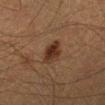| key | value |
|---|---|
| workup | no biopsy performed (imaged during a skin exam) |
| subject | male, aged approximately 40 |
| image-analysis metrics | a symmetry-axis asymmetry near 0.15; an average lesion color of about L≈26 a*≈16 b*≈24 (CIELAB) and a normalized border contrast of about 10; a border-irregularity index near 1.5/10, a within-lesion color-variation index near 3.5/10, and peripheral color asymmetry of about 1.5; an automated nevus-likeness rating near 95 out of 100 and a lesion-detection confidence of about 100/100 |
| tile lighting | cross-polarized |
| location | the left lower leg |
| acquisition | total-body-photography crop, ~15 mm field of view |